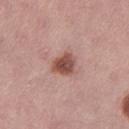Impression:
The lesion was tiled from a total-body skin photograph and was not biopsied.